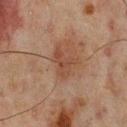Case summary:
* follow-up — catalogued during a skin exam; not biopsied
* lesion diameter — ~4 mm (longest diameter)
* location — the mid back
* patient — male, in their mid-60s
* image source — ~15 mm tile from a whole-body skin photo
* tile lighting — cross-polarized